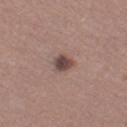Imaged during a routine full-body skin examination; the lesion was not biopsied and no histopathology is available. From the left thigh. This is a white-light tile. A 15 mm crop from a total-body photograph taken for skin-cancer surveillance. A female subject, approximately 35 years of age.